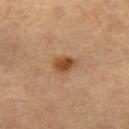Clinical impression:
Captured during whole-body skin photography for melanoma surveillance; the lesion was not biopsied.
Background:
The lesion is located on the leg. A 15 mm close-up extracted from a 3D total-body photography capture. Imaged with cross-polarized lighting. Automated tile analysis of the lesion measured an automated nevus-likeness rating near 95 out of 100 and a lesion-detection confidence of about 100/100. Longest diameter approximately 2.5 mm. A female subject aged 53–57.